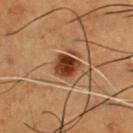| key | value |
|---|---|
| follow-up | imaged on a skin check; not biopsied |
| anatomic site | the front of the torso |
| subject | male, aged around 50 |
| automated metrics | a nevus-likeness score of about 100/100 and a lesion-detection confidence of about 100/100 |
| size | about 3.5 mm |
| imaging modality | 15 mm crop, total-body photography |